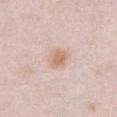Captured during whole-body skin photography for melanoma surveillance; the lesion was not biopsied.
A 15 mm close-up extracted from a 3D total-body photography capture.
Imaged with white-light lighting.
A female patient, in their mid- to late 60s.
The lesion is on the abdomen.
Longest diameter approximately 3 mm.